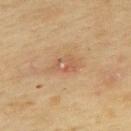Impression: Recorded during total-body skin imaging; not selected for excision or biopsy. Image and clinical context: The tile uses cross-polarized illumination. The recorded lesion diameter is about 2.5 mm. The patient is a female in their 60s. The lesion is on the upper back. A 15 mm crop from a total-body photograph taken for skin-cancer surveillance. The lesion-visualizer software estimated a lesion area of about 3.5 mm² and a shape eccentricity near 0.8. The analysis additionally found a nevus-likeness score of about 0/100 and lesion-presence confidence of about 100/100.